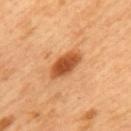<case>
<biopsy_status>not biopsied; imaged during a skin examination</biopsy_status>
</case>